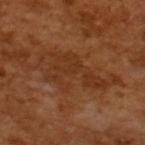| key | value |
|---|---|
| follow-up | total-body-photography surveillance lesion; no biopsy |